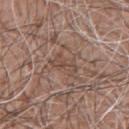Q: Was a biopsy performed?
A: catalogued during a skin exam; not biopsied